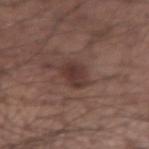Notes:
• follow-up · catalogued during a skin exam; not biopsied
• body site · the left forearm
• subject · male, in their mid- to late 50s
• image-analysis metrics · a lesion–skin lightness drop of about 8 and a normalized lesion–skin contrast near 7.5; a nevus-likeness score of about 60/100 and a detector confidence of about 100 out of 100 that the crop contains a lesion
• acquisition · 15 mm crop, total-body photography
• tile lighting · white-light illumination
• lesion diameter · about 3 mm